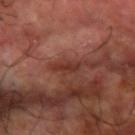follow-up = imaged on a skin check; not biopsied
subject = male, aged approximately 60
size = about 2.5 mm
image = total-body-photography crop, ~15 mm field of view
automated lesion analysis = a border-irregularity rating of about 3.5/10, a within-lesion color-variation index near 0/10, and a peripheral color-asymmetry measure near 0; a classifier nevus-likeness of about 0/100 and a lesion-detection confidence of about 85/100
lighting = cross-polarized
location = the right forearm